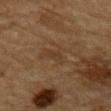Notes:
• notes · no biopsy performed (imaged during a skin exam)
• image-analysis metrics · a lesion area of about 3 mm² and an eccentricity of roughly 0.9; an automated nevus-likeness rating near 0 out of 100
• patient · male, about 85 years old or older
• anatomic site · the mid back
• acquisition · total-body-photography crop, ~15 mm field of view